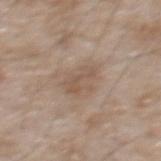From the mid back.
A male subject, aged around 50.
A close-up tile cropped from a whole-body skin photograph, about 15 mm across.
The tile uses white-light illumination.